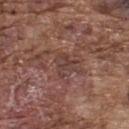Notes:
- notes — no biopsy performed (imaged during a skin exam)
- tile lighting — white-light illumination
- subject — male, about 75 years old
- location — the upper back
- acquisition — ~15 mm tile from a whole-body skin photo